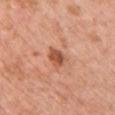biopsy_status: not biopsied; imaged during a skin examination
lesion_size:
  long_diameter_mm_approx: 3.0
lighting: white-light
automated_metrics:
  area_mm2_approx: 5.0
  eccentricity: 0.7
  shape_asymmetry: 0.25
  cielab_L: 54
  cielab_a: 27
  cielab_b: 34
  vs_skin_darker_L: 12.0
  nevus_likeness_0_100: 50
  lesion_detection_confidence_0_100: 100
image:
  source: total-body photography crop
  field_of_view_mm: 15
site: chest
patient:
  sex: female
  age_approx: 50